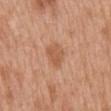A female subject aged 38 to 42.
Captured under white-light illumination.
A lesion tile, about 15 mm wide, cut from a 3D total-body photograph.
On the mid back.